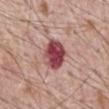workup=catalogued during a skin exam; not biopsied
tile lighting=white-light illumination
acquisition=total-body-photography crop, ~15 mm field of view
diameter=≈4 mm
anatomic site=the abdomen
patient=male, aged 68–72